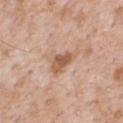Image and clinical context: The lesion is located on the front of the torso. A 15 mm crop from a total-body photograph taken for skin-cancer surveillance. A male subject, aged 63 to 67. An algorithmic analysis of the crop reported an average lesion color of about L≈57 a*≈21 b*≈32 (CIELAB), a lesion–skin lightness drop of about 11, and a normalized lesion–skin contrast near 8. It also reported a border-irregularity rating of about 3/10, a within-lesion color-variation index near 3/10, and peripheral color asymmetry of about 1. The analysis additionally found an automated nevus-likeness rating near 5 out of 100. The tile uses white-light illumination.